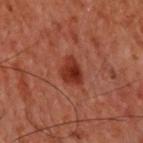<lesion>
  <biopsy_status>not biopsied; imaged during a skin examination</biopsy_status>
  <automated_metrics>
    <area_mm2_approx>6.5</area_mm2_approx>
    <eccentricity>0.65</eccentricity>
    <shape_asymmetry>0.25</shape_asymmetry>
    <border_irregularity_0_10>2.5</border_irregularity_0_10>
    <color_variation_0_10>3.5</color_variation_0_10>
    <peripheral_color_asymmetry>1.5</peripheral_color_asymmetry>
    <nevus_likeness_0_100>95</nevus_likeness_0_100>
    <lesion_detection_confidence_0_100>100</lesion_detection_confidence_0_100>
  </automated_metrics>
  <lighting>cross-polarized</lighting>
  <lesion_size>
    <long_diameter_mm_approx>3.5</long_diameter_mm_approx>
  </lesion_size>
  <site>back</site>
  <image>
    <source>total-body photography crop</source>
    <field_of_view_mm>15</field_of_view_mm>
  </image>
  <patient>
    <sex>male</sex>
    <age_approx>60</age_approx>
  </patient>
</lesion>